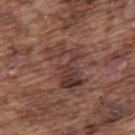Background: A male subject about 75 years old. From the upper back. Cropped from a whole-body photographic skin survey; the tile spans about 15 mm.